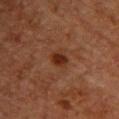* lesion diameter · ~2.5 mm (longest diameter)
* image source · total-body-photography crop, ~15 mm field of view
* tile lighting · cross-polarized
* body site · the upper back
* automated lesion analysis · a lesion area of about 4 mm², an outline eccentricity of about 0.75 (0 = round, 1 = elongated), and a shape-asymmetry score of about 0.2 (0 = symmetric); a within-lesion color-variation index near 2.5/10 and radial color variation of about 1
* patient · female, aged around 70